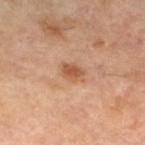Clinical impression: Imaged during a routine full-body skin examination; the lesion was not biopsied and no histopathology is available. Background: Located on the left lower leg. Longest diameter approximately 3 mm. The patient is a female about 60 years old. This image is a 15 mm lesion crop taken from a total-body photograph. Captured under cross-polarized illumination.